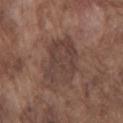Q: Is there a histopathology result?
A: no biopsy performed (imaged during a skin exam)
Q: What is the anatomic site?
A: the front of the torso
Q: What lighting was used for the tile?
A: white-light illumination
Q: How was this image acquired?
A: ~15 mm tile from a whole-body skin photo
Q: What are the patient's age and sex?
A: male, aged 73 to 77